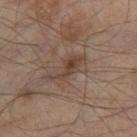Clinical impression: Part of a total-body skin-imaging series; this lesion was reviewed on a skin check and was not flagged for biopsy. Acquisition and patient details: The subject is a male aged 63 to 67. This image is a 15 mm lesion crop taken from a total-body photograph. Located on the right thigh.